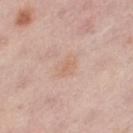<lesion>
<biopsy_status>not biopsied; imaged during a skin examination</biopsy_status>
<lighting>white-light</lighting>
<lesion_size>
  <long_diameter_mm_approx>3.0</long_diameter_mm_approx>
</lesion_size>
<site>left thigh</site>
<automated_metrics>
  <vs_skin_darker_L>5.0</vs_skin_darker_L>
  <vs_skin_contrast_norm>4.5</vs_skin_contrast_norm>
</automated_metrics>
<patient>
  <sex>female</sex>
  <age_approx>45</age_approx>
</patient>
<image>
  <source>total-body photography crop</source>
  <field_of_view_mm>15</field_of_view_mm>
</image>
</lesion>